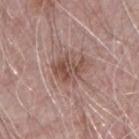Clinical impression:
The lesion was photographed on a routine skin check and not biopsied; there is no pathology result.
Image and clinical context:
Automated image analysis of the tile measured an automated nevus-likeness rating near 30 out of 100. The lesion is located on the right forearm. A male subject, aged 68 to 72. A roughly 15 mm field-of-view crop from a total-body skin photograph. Imaged with white-light lighting. Approximately 4 mm at its widest.